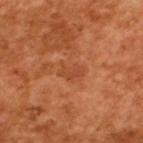Findings:
* workup: no biopsy performed (imaged during a skin exam)
* tile lighting: cross-polarized illumination
* lesion size: ≈3 mm
* image: 15 mm crop, total-body photography
* patient: male, aged 63 to 67